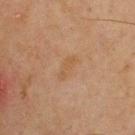follow-up: total-body-photography surveillance lesion; no biopsy
subject: male, in their mid-40s
lesion diameter: ≈3.5 mm
imaging modality: ~15 mm crop, total-body skin-cancer survey
illumination: cross-polarized illumination
body site: the upper back
automated lesion analysis: an average lesion color of about L≈44 a*≈15 b*≈29 (CIELAB) and about 4 CIELAB-L* units darker than the surrounding skin